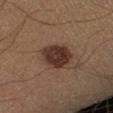Clinical impression:
No biopsy was performed on this lesion — it was imaged during a full skin examination and was not determined to be concerning.
Background:
A roughly 15 mm field-of-view crop from a total-body skin photograph. Located on the left lower leg. A male subject, in their 40s. Imaged with cross-polarized lighting.